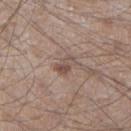Case summary:
- patient · male, aged 53 to 57
- tile lighting · white-light
- site · the right lower leg
- lesion diameter · ~2.5 mm (longest diameter)
- imaging modality · total-body-photography crop, ~15 mm field of view
- automated lesion analysis · a mean CIELAB color near L≈49 a*≈16 b*≈22 and a normalized lesion–skin contrast near 7; a border-irregularity rating of about 4.5/10